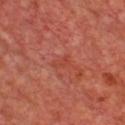Q: Was a biopsy performed?
A: imaged on a skin check; not biopsied
Q: What is the anatomic site?
A: the chest
Q: How large is the lesion?
A: ~2.5 mm (longest diameter)
Q: Automated lesion metrics?
A: an area of roughly 3.5 mm², an eccentricity of roughly 0.8, and a shape-asymmetry score of about 0.35 (0 = symmetric); a nevus-likeness score of about 0/100 and lesion-presence confidence of about 95/100
Q: What lighting was used for the tile?
A: cross-polarized illumination
Q: What kind of image is this?
A: 15 mm crop, total-body photography
Q: What are the patient's age and sex?
A: male, in their mid-60s